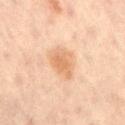The lesion was tiled from a total-body skin photograph and was not biopsied. About 3 mm across. From the right thigh. Cropped from a whole-body photographic skin survey; the tile spans about 15 mm. The subject is a male aged 58 to 62. The tile uses cross-polarized illumination. The lesion-visualizer software estimated a lesion color around L≈65 a*≈21 b*≈36 in CIELAB, roughly 9 lightness units darker than nearby skin, and a normalized border contrast of about 6.5. The software also gave a border-irregularity rating of about 3/10, internal color variation of about 2.5 on a 0–10 scale, and a peripheral color-asymmetry measure near 1.